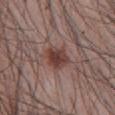The lesion was photographed on a routine skin check and not biopsied; there is no pathology result. On the front of the torso. A lesion tile, about 15 mm wide, cut from a 3D total-body photograph. Automated image analysis of the tile measured a mean CIELAB color near L≈40 a*≈21 b*≈22, a lesion–skin lightness drop of about 11, and a lesion-to-skin contrast of about 9.5 (normalized; higher = more distinct). The analysis additionally found a border-irregularity index near 2.5/10 and a within-lesion color-variation index near 4/10. Captured under white-light illumination. A male subject, about 40 years old.